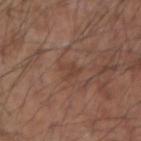Impression:
Captured during whole-body skin photography for melanoma surveillance; the lesion was not biopsied.
Image and clinical context:
Cropped from a whole-body photographic skin survey; the tile spans about 15 mm. The lesion is on the left forearm. The patient is a male in their mid-50s. Measured at roughly 2.5 mm in maximum diameter. Automated image analysis of the tile measured a lesion color around L≈41 a*≈19 b*≈26 in CIELAB, roughly 6 lightness units darker than nearby skin, and a lesion-to-skin contrast of about 5 (normalized; higher = more distinct). It also reported a classifier nevus-likeness of about 5/100 and a detector confidence of about 100 out of 100 that the crop contains a lesion.